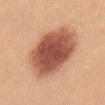biopsy status — catalogued during a skin exam; not biopsied
patient — female, in their mid-20s
image — 15 mm crop, total-body photography
TBP lesion metrics — a lesion area of about 32 mm², an eccentricity of roughly 0.75, and a shape-asymmetry score of about 0.15 (0 = symmetric); a lesion color around L≈53 a*≈26 b*≈31 in CIELAB, roughly 19 lightness units darker than nearby skin, and a lesion-to-skin contrast of about 12 (normalized; higher = more distinct); a color-variation rating of about 5/10
lighting — white-light illumination
anatomic site — the abdomen